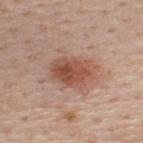follow-up: imaged on a skin check; not biopsied
patient: male, aged 58–62
size: ~5 mm (longest diameter)
acquisition: ~15 mm crop, total-body skin-cancer survey
automated metrics: a mean CIELAB color near L≈51 a*≈23 b*≈29, about 12 CIELAB-L* units darker than the surrounding skin, and a lesion-to-skin contrast of about 8.5 (normalized; higher = more distinct); a border-irregularity rating of about 2/10, internal color variation of about 5.5 on a 0–10 scale, and radial color variation of about 2
tile lighting: white-light
anatomic site: the back